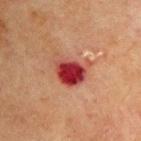Clinical summary:
The lesion is on the upper back. A 15 mm crop from a total-body photograph taken for skin-cancer surveillance. A male subject, aged approximately 70.